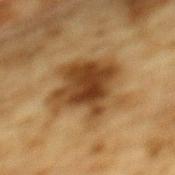– workup: no biopsy performed (imaged during a skin exam)
– acquisition: total-body-photography crop, ~15 mm field of view
– patient: male, approximately 85 years of age or older
– location: the mid back
– size: ≈7 mm
– illumination: cross-polarized illumination